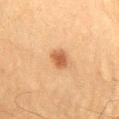workup: total-body-photography surveillance lesion; no biopsy | acquisition: total-body-photography crop, ~15 mm field of view | diameter: about 2.5 mm | subject: male, roughly 60 years of age | tile lighting: cross-polarized illumination | body site: the chest | image-analysis metrics: a lesion area of about 4.5 mm² and a shape eccentricity near 0.6; an average lesion color of about L≈46 a*≈21 b*≈32 (CIELAB), about 10 CIELAB-L* units darker than the surrounding skin, and a normalized border contrast of about 8; a nevus-likeness score of about 100/100 and a lesion-detection confidence of about 100/100.